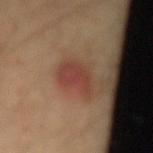Recorded during total-body skin imaging; not selected for excision or biopsy. Automated image analysis of the tile measured border irregularity of about 2.5 on a 0–10 scale and a peripheral color-asymmetry measure near 1. And it measured a lesion-detection confidence of about 100/100. The tile uses cross-polarized illumination. A male patient aged 28–32. The lesion is located on the lower back. A 15 mm close-up extracted from a 3D total-body photography capture.